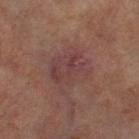biopsy_status: not biopsied; imaged during a skin examination
site: left thigh
lesion_size:
  long_diameter_mm_approx: 5.0
image:
  source: total-body photography crop
  field_of_view_mm: 15
patient:
  sex: female
  age_approx: 60
automated_metrics:
  eccentricity: 0.65
  shape_asymmetry: 0.2
  nevus_likeness_0_100: 0
  lesion_detection_confidence_0_100: 100
lighting: cross-polarized